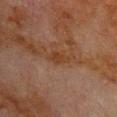<record>
<biopsy_status>not biopsied; imaged during a skin examination</biopsy_status>
<lesion_size>
  <long_diameter_mm_approx>2.5</long_diameter_mm_approx>
</lesion_size>
<image>
  <source>total-body photography crop</source>
  <field_of_view_mm>15</field_of_view_mm>
</image>
<lighting>cross-polarized</lighting>
<patient>
  <sex>male</sex>
  <age_approx>80</age_approx>
</patient>
<site>chest</site>
<automated_metrics>
  <area_mm2_approx>3.0</area_mm2_approx>
  <vs_skin_darker_L>5.0</vs_skin_darker_L>
  <border_irregularity_0_10>2.5</border_irregularity_0_10>
  <color_variation_0_10>1.5</color_variation_0_10>
  <peripheral_color_asymmetry>0.5</peripheral_color_asymmetry>
</automated_metrics>
</record>